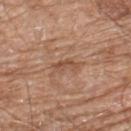Q: Was a biopsy performed?
A: catalogued during a skin exam; not biopsied
Q: Where on the body is the lesion?
A: the upper back
Q: How was this image acquired?
A: ~15 mm crop, total-body skin-cancer survey
Q: Automated lesion metrics?
A: a lesion area of about 3 mm², a shape eccentricity near 0.9, and a symmetry-axis asymmetry near 0.45
Q: What are the patient's age and sex?
A: male, about 80 years old